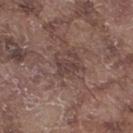lesion diameter: about 3 mm; body site: the right thigh; subject: male, aged 73 to 77; image: ~15 mm tile from a whole-body skin photo; tile lighting: white-light.